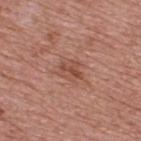Impression: No biopsy was performed on this lesion — it was imaged during a full skin examination and was not determined to be concerning. Image and clinical context: Cropped from a total-body skin-imaging series; the visible field is about 15 mm. The lesion-visualizer software estimated a mean CIELAB color near L≈50 a*≈23 b*≈29, roughly 8 lightness units darker than nearby skin, and a normalized lesion–skin contrast near 6. It also reported a nevus-likeness score of about 5/100 and lesion-presence confidence of about 100/100. The subject is a male aged approximately 70. The lesion is located on the upper back.